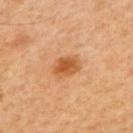Q: What is the imaging modality?
A: ~15 mm tile from a whole-body skin photo
Q: How was the tile lit?
A: cross-polarized illumination
Q: Lesion location?
A: the upper back
Q: How large is the lesion?
A: ~3.5 mm (longest diameter)
Q: Patient demographics?
A: male, roughly 45 years of age